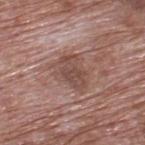{"biopsy_status": "not biopsied; imaged during a skin examination", "site": "upper back", "image": {"source": "total-body photography crop", "field_of_view_mm": 15}, "lesion_size": {"long_diameter_mm_approx": 4.5}, "lighting": "white-light", "patient": {"sex": "male", "age_approx": 70}}